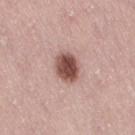The lesion was photographed on a routine skin check and not biopsied; there is no pathology result. A 15 mm crop from a total-body photograph taken for skin-cancer surveillance. On the right thigh. A female subject, aged around 40.